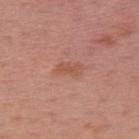Imaged during a routine full-body skin examination; the lesion was not biopsied and no histopathology is available.
The subject is a male approximately 55 years of age.
This image is a 15 mm lesion crop taken from a total-body photograph.
This is a white-light tile.
The lesion's longest dimension is about 3 mm.
The total-body-photography lesion software estimated a lesion area of about 3 mm² and a symmetry-axis asymmetry near 0.4. It also reported a lesion color around L≈53 a*≈25 b*≈30 in CIELAB, roughly 7 lightness units darker than nearby skin, and a lesion-to-skin contrast of about 6 (normalized; higher = more distinct). It also reported a border-irregularity index near 4.5/10 and a color-variation rating of about 0/10.
From the upper back.